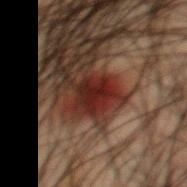biopsy status: no biopsy performed (imaged during a skin exam) | lighting: cross-polarized illumination | automated lesion analysis: an area of roughly 6.5 mm², an eccentricity of roughly 0.35, and a symmetry-axis asymmetry near 0.3; a lesion color around L≈18 a*≈21 b*≈19 in CIELAB, roughly 9 lightness units darker than nearby skin, and a normalized border contrast of about 11.5; an automated nevus-likeness rating near 50 out of 100 and a lesion-detection confidence of about 100/100 | diameter: ~3 mm (longest diameter) | subject: male, aged 48 to 52 | anatomic site: the back | imaging modality: 15 mm crop, total-body photography.